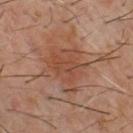Impression:
Imaged during a routine full-body skin examination; the lesion was not biopsied and no histopathology is available.
Image and clinical context:
A 15 mm close-up extracted from a 3D total-body photography capture. Imaged with cross-polarized lighting. From the chest. The subject is a male about 60 years old. Longest diameter approximately 5.5 mm.